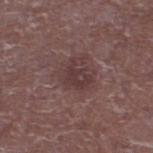No biopsy was performed on this lesion — it was imaged during a full skin examination and was not determined to be concerning.
This is a white-light tile.
Measured at roughly 3 mm in maximum diameter.
Cropped from a whole-body photographic skin survey; the tile spans about 15 mm.
A male subject in their mid- to late 60s.
From the right lower leg.
The total-body-photography lesion software estimated an area of roughly 5.5 mm² and an outline eccentricity of about 0.7 (0 = round, 1 = elongated). It also reported a mean CIELAB color near L≈36 a*≈19 b*≈16 and a lesion–skin lightness drop of about 7.